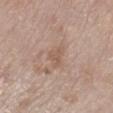A female subject, aged approximately 65. Automated tile analysis of the lesion measured a footprint of about 4.5 mm² and an eccentricity of roughly 0.7. The software also gave a border-irregularity rating of about 3.5/10, internal color variation of about 1.5 on a 0–10 scale, and a peripheral color-asymmetry measure near 0.5. The analysis additionally found a classifier nevus-likeness of about 0/100 and a detector confidence of about 100 out of 100 that the crop contains a lesion. A roughly 15 mm field-of-view crop from a total-body skin photograph. The lesion is located on the right lower leg. This is a white-light tile. The lesion's longest dimension is about 3 mm.